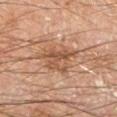Q: Was a biopsy performed?
A: no biopsy performed (imaged during a skin exam)
Q: How was this image acquired?
A: ~15 mm crop, total-body skin-cancer survey
Q: What are the patient's age and sex?
A: male, aged 43–47
Q: How was the tile lit?
A: cross-polarized illumination
Q: How large is the lesion?
A: about 5 mm
Q: Where on the body is the lesion?
A: the arm